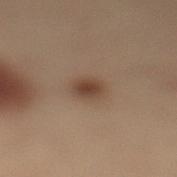The lesion was photographed on a routine skin check and not biopsied; there is no pathology result.
A male patient aged 53 to 57.
This image is a 15 mm lesion crop taken from a total-body photograph.
On the left lower leg.